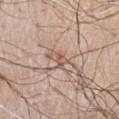Part of a total-body skin-imaging series; this lesion was reviewed on a skin check and was not flagged for biopsy.
A 15 mm close-up tile from a total-body photography series done for melanoma screening.
The recorded lesion diameter is about 2.5 mm.
The lesion is located on the chest.
A male subject in their 70s.
This is a white-light tile.